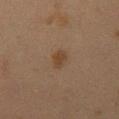Assessment: The lesion was photographed on a routine skin check and not biopsied; there is no pathology result. Background: The lesion's longest dimension is about 3 mm. The total-body-photography lesion software estimated a mean CIELAB color near L≈34 a*≈14 b*≈26, a lesion–skin lightness drop of about 6, and a normalized border contrast of about 7. The software also gave a border-irregularity index near 2/10 and a peripheral color-asymmetry measure near 0.5. The software also gave a lesion-detection confidence of about 100/100. Cropped from a whole-body photographic skin survey; the tile spans about 15 mm. This is a cross-polarized tile. On the right thigh. The subject is a female about 40 years old.